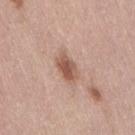workup — imaged on a skin check; not biopsied
image source — ~15 mm tile from a whole-body skin photo
patient — female, aged 38 to 42
tile lighting — white-light illumination
location — the lower back
lesion size — about 3.5 mm